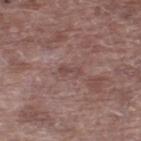Notes:
- workup — no biopsy performed (imaged during a skin exam)
- patient — male, aged 68 to 72
- image source — ~15 mm tile from a whole-body skin photo
- location — the right lower leg
- diameter — about 2.5 mm